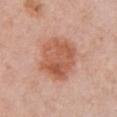{"biopsy_status": "not biopsied; imaged during a skin examination", "lesion_size": {"long_diameter_mm_approx": 5.5}, "image": {"source": "total-body photography crop", "field_of_view_mm": 15}, "patient": {"sex": "female", "age_approx": 50}, "automated_metrics": {"area_mm2_approx": 21.0, "eccentricity": 0.55, "shape_asymmetry": 0.1, "cielab_L": 57, "cielab_a": 25, "cielab_b": 32, "vs_skin_contrast_norm": 8.0, "border_irregularity_0_10": 1.5, "color_variation_0_10": 5.0, "peripheral_color_asymmetry": 1.5}, "lighting": "white-light", "site": "chest"}